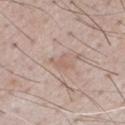A 15 mm close-up tile from a total-body photography series done for melanoma screening. A male patient, aged around 55. Approximately 2.5 mm at its widest.